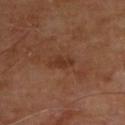Findings:
– illumination · cross-polarized illumination
– patient · male, roughly 70 years of age
– site · the upper back
– acquisition · ~15 mm tile from a whole-body skin photo